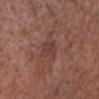Impression: Imaged during a routine full-body skin examination; the lesion was not biopsied and no histopathology is available. Acquisition and patient details: This image is a 15 mm lesion crop taken from a total-body photograph. Located on the chest. The recorded lesion diameter is about 2.5 mm. The patient is a male aged approximately 75. The tile uses white-light illumination.